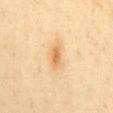  biopsy_status: not biopsied; imaged during a skin examination
  automated_metrics:
    nevus_likeness_0_100: 75
    lesion_detection_confidence_0_100: 100
  lesion_size:
    long_diameter_mm_approx: 3.0
  patient:
    sex: male
    age_approx: 40
  lighting: cross-polarized
  site: mid back
  image:
    source: total-body photography crop
    field_of_view_mm: 15